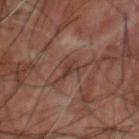Clinical impression:
No biopsy was performed on this lesion — it was imaged during a full skin examination and was not determined to be concerning.
Image and clinical context:
Automated tile analysis of the lesion measured a shape eccentricity near 0.85 and a shape-asymmetry score of about 0.45 (0 = symmetric). The analysis additionally found a lesion color around L≈32 a*≈17 b*≈21 in CIELAB, about 7 CIELAB-L* units darker than the surrounding skin, and a lesion-to-skin contrast of about 7 (normalized; higher = more distinct). The patient is a male aged approximately 60. The tile uses cross-polarized illumination. The lesion's longest dimension is about 2.5 mm. The lesion is located on the upper back. A region of skin cropped from a whole-body photographic capture, roughly 15 mm wide.